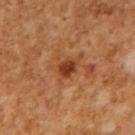The lesion was photographed on a routine skin check and not biopsied; there is no pathology result.
This is a cross-polarized tile.
Approximately 2.5 mm at its widest.
The subject is a male roughly 65 years of age.
A 15 mm crop from a total-body photograph taken for skin-cancer surveillance.